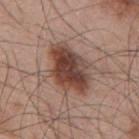{
  "biopsy_status": "not biopsied; imaged during a skin examination",
  "image": {
    "source": "total-body photography crop",
    "field_of_view_mm": 15
  },
  "automated_metrics": {
    "area_mm2_approx": 18.0,
    "eccentricity": 0.8,
    "cielab_L": 43,
    "cielab_a": 20,
    "cielab_b": 25,
    "vs_skin_darker_L": 15.0,
    "vs_skin_contrast_norm": 11.5,
    "color_variation_0_10": 6.5,
    "peripheral_color_asymmetry": 2.0,
    "nevus_likeness_0_100": 95,
    "lesion_detection_confidence_0_100": 100
  },
  "site": "back",
  "lesion_size": {
    "long_diameter_mm_approx": 6.5
  },
  "patient": {
    "sex": "male",
    "age_approx": 55
  },
  "lighting": "white-light"
}